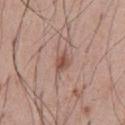follow-up: total-body-photography surveillance lesion; no biopsy | size: ~2.5 mm (longest diameter) | anatomic site: the abdomen | subject: male, approximately 55 years of age | acquisition: ~15 mm tile from a whole-body skin photo.